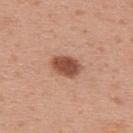<record>
<biopsy_status>not biopsied; imaged during a skin examination</biopsy_status>
<lighting>white-light</lighting>
<site>upper back</site>
<image>
  <source>total-body photography crop</source>
  <field_of_view_mm>15</field_of_view_mm>
</image>
<patient>
  <sex>male</sex>
  <age_approx>30</age_approx>
</patient>
<lesion_size>
  <long_diameter_mm_approx>4.0</long_diameter_mm_approx>
</lesion_size>
</record>